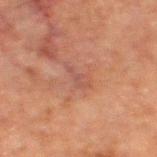This lesion was catalogued during total-body skin photography and was not selected for biopsy.
A male patient aged approximately 80.
Automated tile analysis of the lesion measured a lesion area of about 2.5 mm² and a shape eccentricity near 0.9. And it measured an average lesion color of about L≈40 a*≈20 b*≈23 (CIELAB), roughly 5 lightness units darker than nearby skin, and a normalized border contrast of about 5.
The lesion is on the left thigh.
A lesion tile, about 15 mm wide, cut from a 3D total-body photograph.
This is a cross-polarized tile.